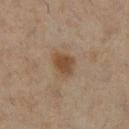workup: total-body-photography surveillance lesion; no biopsy
automated metrics: an area of roughly 6.5 mm² and an outline eccentricity of about 0.6 (0 = round, 1 = elongated); a color-variation rating of about 3/10 and a peripheral color-asymmetry measure near 1; a classifier nevus-likeness of about 95/100
acquisition: total-body-photography crop, ~15 mm field of view
lesion size: ≈3 mm
anatomic site: the right lower leg
subject: female, aged 48–52
tile lighting: cross-polarized illumination